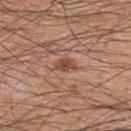| feature | finding |
|---|---|
| follow-up | imaged on a skin check; not biopsied |
| patient | male, in their 60s |
| image source | 15 mm crop, total-body photography |
| location | the upper back |